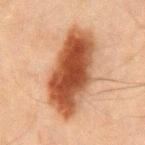The lesion was tiled from a total-body skin photograph and was not biopsied. The subject is a male in their mid-40s. A 15 mm close-up tile from a total-body photography series done for melanoma screening. On the back.